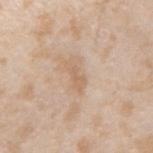Q: Was a biopsy performed?
A: total-body-photography surveillance lesion; no biopsy
Q: Lesion location?
A: the right forearm
Q: Illumination type?
A: white-light illumination
Q: What is the imaging modality?
A: ~15 mm tile from a whole-body skin photo
Q: What are the patient's age and sex?
A: male, in their mid- to late 40s
Q: How large is the lesion?
A: about 3.5 mm